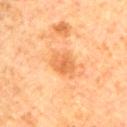A 15 mm crop from a total-body photograph taken for skin-cancer surveillance. The tile uses cross-polarized illumination. An algorithmic analysis of the crop reported a lesion area of about 8.5 mm² and two-axis asymmetry of about 0.2. It also reported an average lesion color of about L≈53 a*≈22 b*≈38 (CIELAB), a lesion–skin lightness drop of about 8, and a normalized border contrast of about 6. It also reported border irregularity of about 2 on a 0–10 scale and a color-variation rating of about 2.5/10. The software also gave a nevus-likeness score of about 25/100. A male subject, aged 78–82. About 4 mm across. The lesion is on the lower back.